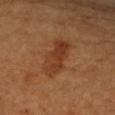Captured during whole-body skin photography for melanoma surveillance; the lesion was not biopsied. A patient aged approximately 70. A 15 mm crop from a total-body photograph taken for skin-cancer surveillance. Captured under cross-polarized illumination. Located on the right upper arm.